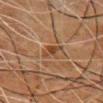follow-up=no biopsy performed (imaged during a skin exam) | subject=male, aged 78–82 | image=~15 mm tile from a whole-body skin photo | body site=the chest.